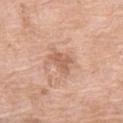biopsy_status: not biopsied; imaged during a skin examination
lighting: white-light
site: left thigh
lesion_size:
  long_diameter_mm_approx: 3.0
patient:
  sex: female
  age_approx: 75
image:
  source: total-body photography crop
  field_of_view_mm: 15